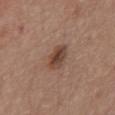A female patient, aged approximately 65. A 15 mm close-up extracted from a 3D total-body photography capture. On the chest.